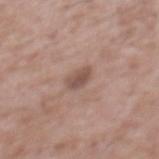follow-up = no biopsy performed (imaged during a skin exam); illumination = white-light illumination; lesion diameter = ≈2.5 mm; body site = the mid back; subject = male, approximately 45 years of age; acquisition = ~15 mm crop, total-body skin-cancer survey.